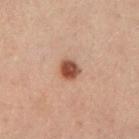The lesion was tiled from a total-body skin photograph and was not biopsied. The lesion's longest dimension is about 2.5 mm. From the left lower leg. A male subject, roughly 55 years of age. A roughly 15 mm field-of-view crop from a total-body skin photograph. The tile uses cross-polarized illumination.